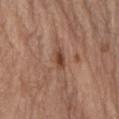Clinical impression:
This lesion was catalogued during total-body skin photography and was not selected for biopsy.
Context:
A region of skin cropped from a whole-body photographic capture, roughly 15 mm wide. The patient is a male in their 70s. Imaged with white-light lighting. The lesion's longest dimension is about 2.5 mm. From the right forearm.